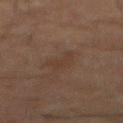The lesion was tiled from a total-body skin photograph and was not biopsied.
The lesion is located on the front of the torso.
The lesion-visualizer software estimated border irregularity of about 6 on a 0–10 scale and radial color variation of about 0.
A male patient, roughly 75 years of age.
Measured at roughly 3 mm in maximum diameter.
A 15 mm close-up tile from a total-body photography series done for melanoma screening.
This is a cross-polarized tile.